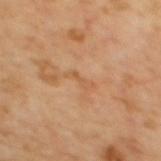Assessment: This lesion was catalogued during total-body skin photography and was not selected for biopsy. Context: The patient is a female roughly 55 years of age. About 3 mm across. The lesion is on the back. Cropped from a whole-body photographic skin survey; the tile spans about 15 mm.